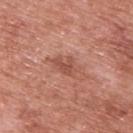notes: no biopsy performed (imaged during a skin exam)
acquisition: ~15 mm tile from a whole-body skin photo
subject: male, aged 68–72
site: the upper back